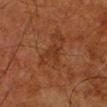Captured during whole-body skin photography for melanoma surveillance; the lesion was not biopsied. Automated image analysis of the tile measured about 5 CIELAB-L* units darker than the surrounding skin and a lesion-to-skin contrast of about 5.5 (normalized; higher = more distinct). The software also gave a border-irregularity index near 4/10. The software also gave a nevus-likeness score of about 0/100 and lesion-presence confidence of about 95/100. The lesion is on the left lower leg. A male patient, about 80 years old. A roughly 15 mm field-of-view crop from a total-body skin photograph. Captured under cross-polarized illumination.